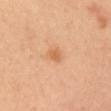Imaged during a routine full-body skin examination; the lesion was not biopsied and no histopathology is available.
Captured under cross-polarized illumination.
The subject is a female approximately 40 years of age.
Cropped from a whole-body photographic skin survey; the tile spans about 15 mm.
On the head or neck.